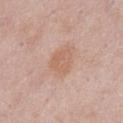Recorded during total-body skin imaging; not selected for excision or biopsy. The lesion is on the front of the torso. A male subject, aged 53–57. The lesion-visualizer software estimated a lesion area of about 7.5 mm², an outline eccentricity of about 0.65 (0 = round, 1 = elongated), and two-axis asymmetry of about 0.3. The software also gave border irregularity of about 2.5 on a 0–10 scale and peripheral color asymmetry of about 1. A region of skin cropped from a whole-body photographic capture, roughly 15 mm wide. Captured under white-light illumination.